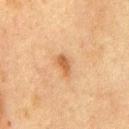Recorded during total-body skin imaging; not selected for excision or biopsy.
Longest diameter approximately 2.5 mm.
The lesion is located on the chest.
Captured under cross-polarized illumination.
A roughly 15 mm field-of-view crop from a total-body skin photograph.
The subject is a male aged 73–77.
The total-body-photography lesion software estimated a classifier nevus-likeness of about 60/100 and a detector confidence of about 100 out of 100 that the crop contains a lesion.